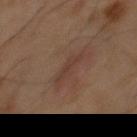{"biopsy_status": "not biopsied; imaged during a skin examination", "lighting": "cross-polarized", "patient": {"sex": "male", "age_approx": 60}, "image": {"source": "total-body photography crop", "field_of_view_mm": 15}, "site": "front of the torso"}